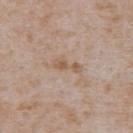Q: Was this lesion biopsied?
A: catalogued during a skin exam; not biopsied
Q: What kind of image is this?
A: 15 mm crop, total-body photography
Q: Who is the patient?
A: male, approximately 65 years of age
Q: Automated lesion metrics?
A: a lesion color around L≈58 a*≈16 b*≈28 in CIELAB, about 7 CIELAB-L* units darker than the surrounding skin, and a normalized border contrast of about 5.5; a border-irregularity index near 8/10 and peripheral color asymmetry of about 0.5; an automated nevus-likeness rating near 0 out of 100
Q: Lesion size?
A: about 5 mm
Q: Illumination type?
A: white-light
Q: Where on the body is the lesion?
A: the abdomen